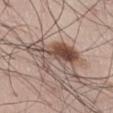Q: Is there a histopathology result?
A: no biopsy performed (imaged during a skin exam)
Q: Who is the patient?
A: male, in their 70s
Q: What is the imaging modality?
A: ~15 mm tile from a whole-body skin photo
Q: Lesion location?
A: the front of the torso